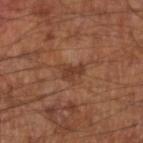Impression:
The lesion was photographed on a routine skin check and not biopsied; there is no pathology result.
Clinical summary:
A male patient aged around 65. The total-body-photography lesion software estimated a mean CIELAB color near L≈36 a*≈21 b*≈28 and a normalized lesion–skin contrast near 7. The analysis additionally found an automated nevus-likeness rating near 15 out of 100. Cropped from a whole-body photographic skin survey; the tile spans about 15 mm. Imaged with cross-polarized lighting. From the left arm. The recorded lesion diameter is about 2.5 mm.